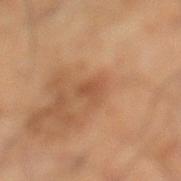Recorded during total-body skin imaging; not selected for excision or biopsy.
The lesion is located on the leg.
About 3 mm across.
The tile uses cross-polarized illumination.
The subject is a male approximately 60 years of age.
Cropped from a total-body skin-imaging series; the visible field is about 15 mm.